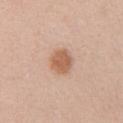Captured during whole-body skin photography for melanoma surveillance; the lesion was not biopsied.
A female patient, aged 38–42.
The lesion is on the chest.
A lesion tile, about 15 mm wide, cut from a 3D total-body photograph.